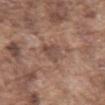biopsy status=imaged on a skin check; not biopsied
patient=male, approximately 75 years of age
acquisition=15 mm crop, total-body photography
site=the abdomen